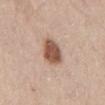Impression:
Captured during whole-body skin photography for melanoma surveillance; the lesion was not biopsied.
Acquisition and patient details:
A male subject aged approximately 60. The lesion is on the mid back. This image is a 15 mm lesion crop taken from a total-body photograph.